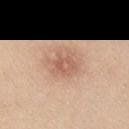Captured during whole-body skin photography for melanoma surveillance; the lesion was not biopsied.